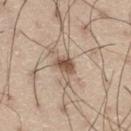Case summary:
– subject: male, roughly 55 years of age
– image: ~15 mm tile from a whole-body skin photo
– automated metrics: a border-irregularity index near 2.5/10, a color-variation rating of about 3/10, and peripheral color asymmetry of about 1; an automated nevus-likeness rating near 95 out of 100 and a lesion-detection confidence of about 100/100
– lighting: white-light illumination
– anatomic site: the right thigh